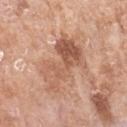Part of a total-body skin-imaging series; this lesion was reviewed on a skin check and was not flagged for biopsy.
Located on the left forearm.
A female subject aged 73 to 77.
A 15 mm close-up extracted from a 3D total-body photography capture.